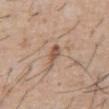workup = no biopsy performed (imaged during a skin exam)
tile lighting = white-light illumination
imaging modality = 15 mm crop, total-body photography
site = the front of the torso
lesion size = ~3 mm (longest diameter)
subject = male, about 55 years old